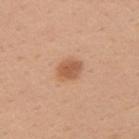Clinical impression:
The lesion was photographed on a routine skin check and not biopsied; there is no pathology result.
Clinical summary:
About 3 mm across. The tile uses white-light illumination. Cropped from a total-body skin-imaging series; the visible field is about 15 mm. A female subject aged around 30. On the left upper arm. An algorithmic analysis of the crop reported an area of roughly 5 mm², an eccentricity of roughly 0.55, and two-axis asymmetry of about 0.2. The analysis additionally found roughly 11 lightness units darker than nearby skin and a normalized border contrast of about 7.5.